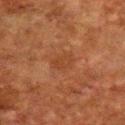| field | value |
|---|---|
| notes | no biopsy performed (imaged during a skin exam) |
| site | the front of the torso |
| imaging modality | ~15 mm crop, total-body skin-cancer survey |
| subject | male, approximately 80 years of age |
| illumination | cross-polarized |
| lesion size | about 3 mm |
| automated lesion analysis | an outline eccentricity of about 0.65 (0 = round, 1 = elongated) and two-axis asymmetry of about 0.2; a lesion color around L≈33 a*≈21 b*≈29 in CIELAB, about 5 CIELAB-L* units darker than the surrounding skin, and a normalized border contrast of about 4.5; an automated nevus-likeness rating near 0 out of 100 and a lesion-detection confidence of about 100/100 |